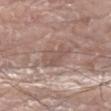follow-up: total-body-photography surveillance lesion; no biopsy | image: ~15 mm tile from a whole-body skin photo | site: the right lower leg | illumination: white-light | TBP lesion metrics: a shape eccentricity near 0.75 and a shape-asymmetry score of about 0.35 (0 = symmetric); an average lesion color of about L≈54 a*≈17 b*≈23 (CIELAB), roughly 7 lightness units darker than nearby skin, and a normalized lesion–skin contrast near 5; a border-irregularity index near 4/10 and internal color variation of about 2.5 on a 0–10 scale; a nevus-likeness score of about 0/100 and a detector confidence of about 70 out of 100 that the crop contains a lesion | subject: male, aged approximately 80.